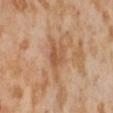| key | value |
|---|---|
| follow-up | total-body-photography surveillance lesion; no biopsy |
| lesion diameter | ~4 mm (longest diameter) |
| lighting | cross-polarized illumination |
| image source | ~15 mm tile from a whole-body skin photo |
| subject | female, in their mid- to late 50s |
| site | the abdomen |